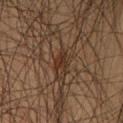Part of a total-body skin-imaging series; this lesion was reviewed on a skin check and was not flagged for biopsy.
The subject is a male in their mid-40s.
Captured under cross-polarized illumination.
Longest diameter approximately 2.5 mm.
A region of skin cropped from a whole-body photographic capture, roughly 15 mm wide.
The total-body-photography lesion software estimated a lesion area of about 3.5 mm² and an outline eccentricity of about 0.75 (0 = round, 1 = elongated).
From the left thigh.